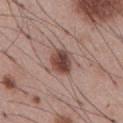Q: Was this lesion biopsied?
A: imaged on a skin check; not biopsied
Q: What kind of image is this?
A: total-body-photography crop, ~15 mm field of view
Q: Lesion location?
A: the abdomen
Q: Who is the patient?
A: male, aged around 55